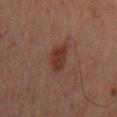  lighting: cross-polarized
  site: abdomen
  patient:
    sex: male
    age_approx: 65
  lesion_size:
    long_diameter_mm_approx: 4.0
  image:
    source: total-body photography crop
    field_of_view_mm: 15
  automated_metrics:
    cielab_L: 27
    cielab_a: 18
    cielab_b: 22
    vs_skin_darker_L: 7.0
    vs_skin_contrast_norm: 8.5
    nevus_likeness_0_100: 90
    lesion_detection_confidence_0_100: 100